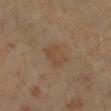Assessment:
Imaged during a routine full-body skin examination; the lesion was not biopsied and no histopathology is available.
Context:
The patient is a male about 45 years old. The lesion is on the leg. This is a cross-polarized tile. A 15 mm close-up tile from a total-body photography series done for melanoma screening.